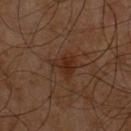Notes:
- follow-up · total-body-photography surveillance lesion; no biopsy
- location · the chest
- subject · male, approximately 60 years of age
- diameter · about 3 mm
- imaging modality · ~15 mm crop, total-body skin-cancer survey
- automated lesion analysis · an area of roughly 6 mm², a shape eccentricity near 0.4, and two-axis asymmetry of about 0.45; an average lesion color of about L≈25 a*≈18 b*≈25 (CIELAB), about 6 CIELAB-L* units darker than the surrounding skin, and a normalized border contrast of about 7.5; a border-irregularity index near 4.5/10, internal color variation of about 3 on a 0–10 scale, and a peripheral color-asymmetry measure near 1
- illumination · cross-polarized